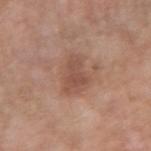The lesion was photographed on a routine skin check and not biopsied; there is no pathology result.
On the left forearm.
A roughly 15 mm field-of-view crop from a total-body skin photograph.
A female subject, aged 53 to 57.